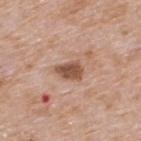Impression: The lesion was photographed on a routine skin check and not biopsied; there is no pathology result. Background: The patient is a male aged around 65. Located on the upper back. Approximately 3 mm at its widest. Cropped from a whole-body photographic skin survey; the tile spans about 15 mm.